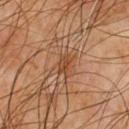follow-up = no biopsy performed (imaged during a skin exam); diameter = ≈2.5 mm; site = the chest; tile lighting = cross-polarized illumination; image = ~15 mm crop, total-body skin-cancer survey; patient = male, in their mid-60s.